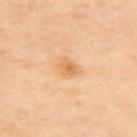Cropped from a total-body skin-imaging series; the visible field is about 15 mm. Automated image analysis of the tile measured an area of roughly 4 mm², a shape eccentricity near 0.7, and two-axis asymmetry of about 0.3. The analysis additionally found an average lesion color of about L≈69 a*≈22 b*≈43 (CIELAB), a lesion–skin lightness drop of about 9, and a normalized lesion–skin contrast near 6. And it measured border irregularity of about 2.5 on a 0–10 scale, internal color variation of about 3 on a 0–10 scale, and radial color variation of about 1. And it measured a classifier nevus-likeness of about 10/100 and a detector confidence of about 100 out of 100 that the crop contains a lesion. The recorded lesion diameter is about 2.5 mm. This is a cross-polarized tile. The patient is a female in their mid-40s. Located on the upper back.